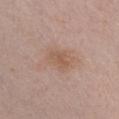biopsy_status: not biopsied; imaged during a skin examination
image:
  source: total-body photography crop
  field_of_view_mm: 15
patient:
  sex: female
  age_approx: 20
lesion_size:
  long_diameter_mm_approx: 2.5
site: chest
lighting: white-light